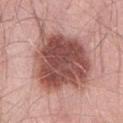No biopsy was performed on this lesion — it was imaged during a full skin examination and was not determined to be concerning. From the abdomen. A 15 mm crop from a total-body photograph taken for skin-cancer surveillance. A female patient in their mid- to late 60s.